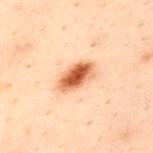Imaged during a routine full-body skin examination; the lesion was not biopsied and no histopathology is available.
About 4.5 mm across.
The subject is a male about 30 years old.
A lesion tile, about 15 mm wide, cut from a 3D total-body photograph.
On the upper back.